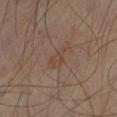Notes:
• notes: total-body-photography surveillance lesion; no biopsy
• automated lesion analysis: an eccentricity of roughly 0.9 and a shape-asymmetry score of about 0.4 (0 = symmetric); a nevus-likeness score of about 0/100 and lesion-presence confidence of about 100/100
• image source: 15 mm crop, total-body photography
• diameter: about 4 mm
• subject: approximately 55 years of age
• site: the leg